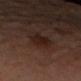This lesion was catalogued during total-body skin photography and was not selected for biopsy.
The patient is a female aged approximately 65.
About 3 mm across.
On the right forearm.
The tile uses cross-polarized illumination.
A 15 mm close-up extracted from a 3D total-body photography capture.